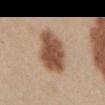Impression: The lesion was tiled from a total-body skin photograph and was not biopsied. Background: A 15 mm close-up tile from a total-body photography series done for melanoma screening. Approximately 6.5 mm at its widest. A male subject roughly 30 years of age. The lesion is located on the abdomen.